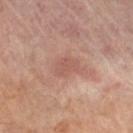Findings:
* follow-up — imaged on a skin check; not biopsied
* automated lesion analysis — an average lesion color of about L≈53 a*≈22 b*≈25 (CIELAB), about 7 CIELAB-L* units darker than the surrounding skin, and a normalized border contrast of about 5; a border-irregularity index near 3/10, internal color variation of about 1.5 on a 0–10 scale, and a peripheral color-asymmetry measure near 0.5; a classifier nevus-likeness of about 0/100 and a lesion-detection confidence of about 100/100
* body site — the right thigh
* patient — male, aged 68–72
* image — ~15 mm crop, total-body skin-cancer survey
* tile lighting — cross-polarized illumination
* lesion size — ~3 mm (longest diameter)